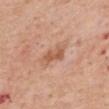{
  "biopsy_status": "not biopsied; imaged during a skin examination",
  "automated_metrics": {
    "area_mm2_approx": 4.5,
    "eccentricity": 0.8,
    "shape_asymmetry": 0.3,
    "cielab_L": 57,
    "cielab_a": 23,
    "cielab_b": 33,
    "vs_skin_darker_L": 10.0,
    "vs_skin_contrast_norm": 7.0,
    "border_irregularity_0_10": 3.0,
    "color_variation_0_10": 2.0,
    "peripheral_color_asymmetry": 1.0
  },
  "lesion_size": {
    "long_diameter_mm_approx": 3.0
  },
  "image": {
    "source": "total-body photography crop",
    "field_of_view_mm": 15
  },
  "lighting": "white-light",
  "patient": {
    "sex": "male",
    "age_approx": 65
  },
  "site": "mid back"
}